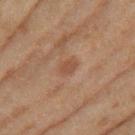The lesion was tiled from a total-body skin photograph and was not biopsied. Imaged with cross-polarized lighting. Cropped from a total-body skin-imaging series; the visible field is about 15 mm. An algorithmic analysis of the crop reported an area of roughly 3.5 mm², an outline eccentricity of about 0.7 (0 = round, 1 = elongated), and two-axis asymmetry of about 0.25. The analysis additionally found a border-irregularity rating of about 2/10, a color-variation rating of about 1/10, and peripheral color asymmetry of about 0.5. It also reported a nevus-likeness score of about 0/100 and lesion-presence confidence of about 100/100. The lesion is located on the leg. The lesion's longest dimension is about 2.5 mm. The patient is a female about 60 years old.